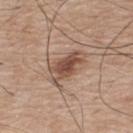workup: catalogued during a skin exam; not biopsied | lesion size: ~5 mm (longest diameter) | image: total-body-photography crop, ~15 mm field of view | subject: male, in their mid-70s | site: the upper back | image-analysis metrics: a mean CIELAB color near L≈50 a*≈19 b*≈27 and a normalized border contrast of about 8.5; a border-irregularity rating of about 3.5/10, a color-variation rating of about 5/10, and radial color variation of about 1.5; an automated nevus-likeness rating near 90 out of 100 and lesion-presence confidence of about 100/100.